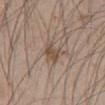Automated tile analysis of the lesion measured an automated nevus-likeness rating near 5 out of 100 and a detector confidence of about 100 out of 100 that the crop contains a lesion. A region of skin cropped from a whole-body photographic capture, roughly 15 mm wide. The lesion's longest dimension is about 2.5 mm. A male subject, approximately 45 years of age. The lesion is located on the abdomen. Imaged with white-light lighting.